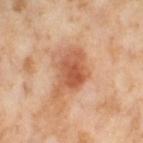Captured during whole-body skin photography for melanoma surveillance; the lesion was not biopsied.
On the right thigh.
A female subject approximately 55 years of age.
A 15 mm close-up tile from a total-body photography series done for melanoma screening.
The total-body-photography lesion software estimated a within-lesion color-variation index near 5/10 and a peripheral color-asymmetry measure near 1.5.
Measured at roughly 5.5 mm in maximum diameter.